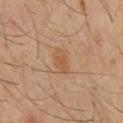Impression: No biopsy was performed on this lesion — it was imaged during a full skin examination and was not determined to be concerning. Context: A male patient approximately 60 years of age. A 15 mm close-up extracted from a 3D total-body photography capture. Measured at roughly 3.5 mm in maximum diameter. Automated image analysis of the tile measured a footprint of about 4.5 mm², a shape eccentricity near 0.85, and two-axis asymmetry of about 0.3. The analysis additionally found about 7 CIELAB-L* units darker than the surrounding skin and a lesion-to-skin contrast of about 6 (normalized; higher = more distinct). And it measured a nevus-likeness score of about 15/100 and lesion-presence confidence of about 100/100. On the chest. Captured under cross-polarized illumination.